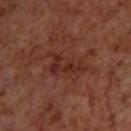notes: catalogued during a skin exam; not biopsied
lighting: cross-polarized illumination
TBP lesion metrics: a footprint of about 7 mm², a shape eccentricity near 0.9, and a symmetry-axis asymmetry near 0.5; a mean CIELAB color near L≈27 a*≈22 b*≈25, roughly 6 lightness units darker than nearby skin, and a lesion-to-skin contrast of about 6.5 (normalized; higher = more distinct); a classifier nevus-likeness of about 0/100 and a lesion-detection confidence of about 100/100
image source: ~15 mm tile from a whole-body skin photo
subject: male, about 70 years old
location: the upper back
size: ≈4.5 mm